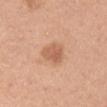Recorded during total-body skin imaging; not selected for excision or biopsy.
Approximately 3 mm at its widest.
This is a white-light tile.
Located on the right upper arm.
A 15 mm crop from a total-body photograph taken for skin-cancer surveillance.
A male patient, in their 30s.